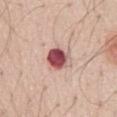Q: Was a biopsy performed?
A: no biopsy performed (imaged during a skin exam)
Q: What is the imaging modality?
A: total-body-photography crop, ~15 mm field of view
Q: What are the patient's age and sex?
A: male, aged around 70
Q: Where on the body is the lesion?
A: the abdomen
Q: How was the tile lit?
A: white-light
Q: How large is the lesion?
A: about 3 mm